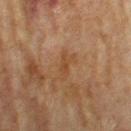  biopsy_status: not biopsied; imaged during a skin examination